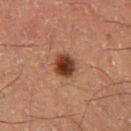follow-up=no biopsy performed (imaged during a skin exam) | image=total-body-photography crop, ~15 mm field of view | patient=male, aged 58–62 | illumination=cross-polarized illumination | site=the left thigh.